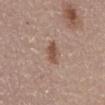Impression: No biopsy was performed on this lesion — it was imaged during a full skin examination and was not determined to be concerning. Acquisition and patient details: A close-up tile cropped from a whole-body skin photograph, about 15 mm across. The patient is a female in their mid-60s. Automated image analysis of the tile measured a mean CIELAB color near L≈51 a*≈19 b*≈27 and a normalized border contrast of about 7.5. The lesion is located on the abdomen. Measured at roughly 3 mm in maximum diameter. Imaged with white-light lighting.